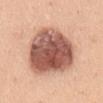Q: Was a biopsy performed?
A: imaged on a skin check; not biopsied
Q: What is the imaging modality?
A: 15 mm crop, total-body photography
Q: Lesion location?
A: the abdomen
Q: What did automated image analysis measure?
A: a shape eccentricity near 0.6; an average lesion color of about L≈61 a*≈22 b*≈29 (CIELAB) and a normalized border contrast of about 9.5
Q: What are the patient's age and sex?
A: male, in their mid- to late 30s
Q: Illumination type?
A: white-light illumination